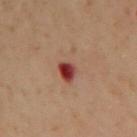{
  "biopsy_status": "not biopsied; imaged during a skin examination",
  "patient": {
    "sex": "female",
    "age_approx": 60
  },
  "site": "upper back",
  "lesion_size": {
    "long_diameter_mm_approx": 4.0
  },
  "image": {
    "source": "total-body photography crop",
    "field_of_view_mm": 15
  },
  "lighting": "cross-polarized"
}